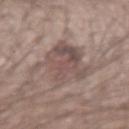size — ≈6.5 mm; subject — male, aged 38–42; site — the right forearm; image — total-body-photography crop, ~15 mm field of view; tile lighting — white-light illumination.